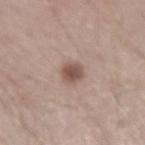biopsy status: total-body-photography surveillance lesion; no biopsy
body site: the right forearm
patient: male, aged 43 to 47
tile lighting: white-light
automated metrics: a lesion area of about 5 mm², an outline eccentricity of about 0.7 (0 = round, 1 = elongated), and a shape-asymmetry score of about 0.2 (0 = symmetric); border irregularity of about 1.5 on a 0–10 scale and internal color variation of about 3 on a 0–10 scale; a nevus-likeness score of about 90/100 and lesion-presence confidence of about 100/100
image source: ~15 mm tile from a whole-body skin photo